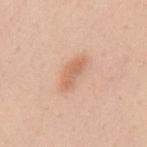{"patient": {"sex": "female", "age_approx": 40}, "site": "mid back", "image": {"source": "total-body photography crop", "field_of_view_mm": 15}, "lighting": "white-light", "automated_metrics": {"area_mm2_approx": 6.0, "eccentricity": 0.9, "shape_asymmetry": 0.3, "border_irregularity_0_10": 3.5, "color_variation_0_10": 1.5, "peripheral_color_asymmetry": 0.5, "nevus_likeness_0_100": 75, "lesion_detection_confidence_0_100": 100}}